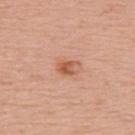body site — the upper back | imaging modality — ~15 mm crop, total-body skin-cancer survey | subject — female, approximately 50 years of age | lesion diameter — ~2.5 mm (longest diameter) | image-analysis metrics — border irregularity of about 3 on a 0–10 scale, a color-variation rating of about 4/10, and radial color variation of about 1; a lesion-detection confidence of about 100/100 | illumination — white-light illumination.